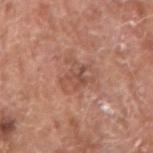notes: catalogued during a skin exam; not biopsied
automated metrics: a lesion area of about 7.5 mm² and an outline eccentricity of about 0.55 (0 = round, 1 = elongated); a mean CIELAB color near L≈51 a*≈23 b*≈28 and a lesion-to-skin contrast of about 5.5 (normalized; higher = more distinct)
body site: the right upper arm
illumination: white-light illumination
image: 15 mm crop, total-body photography
patient: male, roughly 65 years of age
lesion diameter: ≈3.5 mm